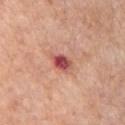Acquisition and patient details: This is a white-light tile. The lesion is located on the front of the torso. A male patient, in their 60s. Approximately 3 mm at its widest. Automated tile analysis of the lesion measured a footprint of about 4.5 mm². It also reported a lesion color around L≈51 a*≈33 b*≈26 in CIELAB and a normalized border contrast of about 10.5. The analysis additionally found a within-lesion color-variation index near 7.5/10 and radial color variation of about 3. And it measured an automated nevus-likeness rating near 0 out of 100 and a lesion-detection confidence of about 100/100. A region of skin cropped from a whole-body photographic capture, roughly 15 mm wide.